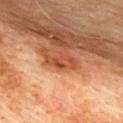Clinical impression: No biopsy was performed on this lesion — it was imaged during a full skin examination and was not determined to be concerning. Image and clinical context: A male patient, roughly 75 years of age. The lesion's longest dimension is about 4 mm. On the upper back. This is a cross-polarized tile. A roughly 15 mm field-of-view crop from a total-body skin photograph.